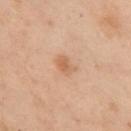Clinical impression: Part of a total-body skin-imaging series; this lesion was reviewed on a skin check and was not flagged for biopsy. Background: Cropped from a total-body skin-imaging series; the visible field is about 15 mm. Captured under cross-polarized illumination. A female patient, approximately 55 years of age. The lesion is on the chest. Longest diameter approximately 2.5 mm. An algorithmic analysis of the crop reported an average lesion color of about L≈51 a*≈18 b*≈30 (CIELAB), a lesion–skin lightness drop of about 7, and a lesion-to-skin contrast of about 6 (normalized; higher = more distinct). The software also gave border irregularity of about 2 on a 0–10 scale, a color-variation rating of about 2/10, and peripheral color asymmetry of about 0.5. The software also gave a nevus-likeness score of about 50/100 and a lesion-detection confidence of about 100/100.